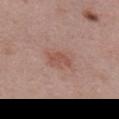Part of a total-body skin-imaging series; this lesion was reviewed on a skin check and was not flagged for biopsy.
A close-up tile cropped from a whole-body skin photograph, about 15 mm across.
The lesion is on the chest.
The lesion-visualizer software estimated an area of roughly 4 mm² and an outline eccentricity of about 0.8 (0 = round, 1 = elongated). The analysis additionally found a mean CIELAB color near L≈52 a*≈23 b*≈26 and a lesion-to-skin contrast of about 6 (normalized; higher = more distinct).
The subject is a female in their 50s.
The lesion's longest dimension is about 3 mm.
Captured under white-light illumination.